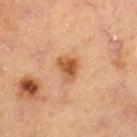The lesion was photographed on a routine skin check and not biopsied; there is no pathology result.
The recorded lesion diameter is about 3 mm.
A male subject, aged approximately 65.
This is a cross-polarized tile.
Located on the leg.
A 15 mm crop from a total-body photograph taken for skin-cancer surveillance.